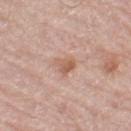Impression: The lesion was photographed on a routine skin check and not biopsied; there is no pathology result. Acquisition and patient details: A male patient about 70 years old. The lesion is on the left thigh. The recorded lesion diameter is about 2.5 mm. Imaged with white-light lighting. A roughly 15 mm field-of-view crop from a total-body skin photograph.